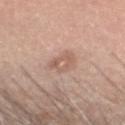– biopsy status — imaged on a skin check; not biopsied
– body site — the head or neck
– image — ~15 mm tile from a whole-body skin photo
– tile lighting — white-light illumination
– subject — male, about 60 years old
– size — ~2.5 mm (longest diameter)